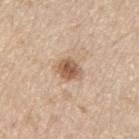Q: Was this lesion biopsied?
A: no biopsy performed (imaged during a skin exam)
Q: How was the tile lit?
A: white-light
Q: What is the imaging modality?
A: ~15 mm crop, total-body skin-cancer survey
Q: Where on the body is the lesion?
A: the left thigh
Q: Who is the patient?
A: male, aged approximately 70
Q: What is the lesion's diameter?
A: about 3 mm